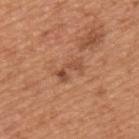Impression:
Part of a total-body skin-imaging series; this lesion was reviewed on a skin check and was not flagged for biopsy.
Image and clinical context:
Cropped from a whole-body photographic skin survey; the tile spans about 15 mm. The lesion is located on the left upper arm. A male patient, aged 63–67.